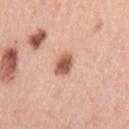{"biopsy_status": "not biopsied; imaged during a skin examination", "image": {"source": "total-body photography crop", "field_of_view_mm": 15}, "automated_metrics": {"cielab_L": 58, "cielab_a": 23, "cielab_b": 30, "vs_skin_darker_L": 16.0, "vs_skin_contrast_norm": 10.0, "border_irregularity_0_10": 1.5, "color_variation_0_10": 4.0, "peripheral_color_asymmetry": 1.0, "nevus_likeness_0_100": 95, "lesion_detection_confidence_0_100": 100}, "site": "left upper arm", "lesion_size": {"long_diameter_mm_approx": 2.5}, "patient": {"sex": "female", "age_approx": 60}}